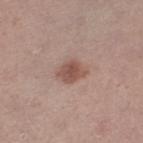notes: imaged on a skin check; not biopsied
imaging modality: total-body-photography crop, ~15 mm field of view
TBP lesion metrics: a symmetry-axis asymmetry near 0.15; roughly 10 lightness units darker than nearby skin and a lesion-to-skin contrast of about 7.5 (normalized; higher = more distinct); border irregularity of about 2 on a 0–10 scale and internal color variation of about 2.5 on a 0–10 scale; a classifier nevus-likeness of about 85/100 and a lesion-detection confidence of about 100/100
body site: the left thigh
size: about 2.5 mm
subject: female, aged around 50